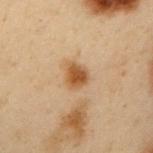{
  "biopsy_status": "not biopsied; imaged during a skin examination",
  "automated_metrics": {
    "cielab_L": 44,
    "cielab_a": 17,
    "cielab_b": 32,
    "vs_skin_darker_L": 11.0,
    "vs_skin_contrast_norm": 9.5,
    "border_irregularity_0_10": 2.5,
    "color_variation_0_10": 3.0,
    "peripheral_color_asymmetry": 1.0,
    "nevus_likeness_0_100": 95,
    "lesion_detection_confidence_0_100": 100
  },
  "lighting": "cross-polarized",
  "site": "left upper arm",
  "patient": {
    "sex": "male",
    "age_approx": 55
  },
  "image": {
    "source": "total-body photography crop",
    "field_of_view_mm": 15
  },
  "lesion_size": {
    "long_diameter_mm_approx": 3.0
  }
}